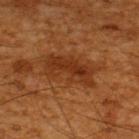notes: imaged on a skin check; not biopsied | imaging modality: ~15 mm crop, total-body skin-cancer survey | patient: male, about 65 years old | anatomic site: the upper back.